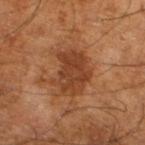The lesion was tiled from a total-body skin photograph and was not biopsied.
Located on the left lower leg.
An algorithmic analysis of the crop reported a mean CIELAB color near L≈40 a*≈23 b*≈34 and a normalized lesion–skin contrast near 8. The analysis additionally found a border-irregularity index near 4/10 and a peripheral color-asymmetry measure near 1.
A male patient roughly 65 years of age.
Cropped from a whole-body photographic skin survey; the tile spans about 15 mm.
Captured under cross-polarized illumination.